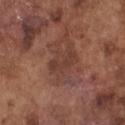| key | value |
|---|---|
| biopsy status | imaged on a skin check; not biopsied |
| automated lesion analysis | a lesion–skin lightness drop of about 6 and a normalized border contrast of about 5.5 |
| lesion diameter | ≈3.5 mm |
| tile lighting | white-light illumination |
| site | the chest |
| patient | male, about 75 years old |
| acquisition | ~15 mm crop, total-body skin-cancer survey |